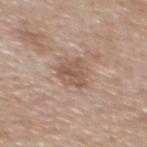This lesion was catalogued during total-body skin photography and was not selected for biopsy. Longest diameter approximately 3.5 mm. An algorithmic analysis of the crop reported a lesion color around L≈55 a*≈18 b*≈27 in CIELAB, about 9 CIELAB-L* units darker than the surrounding skin, and a lesion-to-skin contrast of about 6.5 (normalized; higher = more distinct). A 15 mm close-up extracted from a 3D total-body photography capture. The tile uses white-light illumination. Located on the back. A female subject roughly 40 years of age.